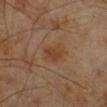Recorded during total-body skin imaging; not selected for excision or biopsy. The patient is a male aged 68 to 72. Located on the right lower leg. A roughly 15 mm field-of-view crop from a total-body skin photograph. The recorded lesion diameter is about 3.5 mm. The tile uses cross-polarized illumination.